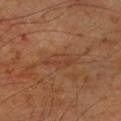Imaged during a routine full-body skin examination; the lesion was not biopsied and no histopathology is available. Measured at roughly 4 mm in maximum diameter. Located on the left forearm. A 15 mm close-up tile from a total-body photography series done for melanoma screening. A male subject aged around 60. Automated image analysis of the tile measured border irregularity of about 3 on a 0–10 scale, a within-lesion color-variation index near 2/10, and radial color variation of about 0.5.